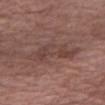Clinical impression: Imaged during a routine full-body skin examination; the lesion was not biopsied and no histopathology is available. Image and clinical context: Located on the right thigh. Cropped from a whole-body photographic skin survey; the tile spans about 15 mm. A male subject aged 78–82. Approximately 6.5 mm at its widest.